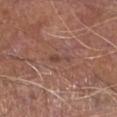Captured during whole-body skin photography for melanoma surveillance; the lesion was not biopsied.
This is a white-light tile.
The patient is a male in their mid- to late 60s.
A close-up tile cropped from a whole-body skin photograph, about 15 mm across.
The lesion-visualizer software estimated a mean CIELAB color near L≈44 a*≈19 b*≈24, roughly 7 lightness units darker than nearby skin, and a lesion-to-skin contrast of about 5.5 (normalized; higher = more distinct). The software also gave a border-irregularity index near 4.5/10, a color-variation rating of about 0/10, and peripheral color asymmetry of about 0.
From the left lower leg.
The recorded lesion diameter is about 3 mm.